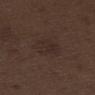Q: Was a biopsy performed?
A: imaged on a skin check; not biopsied
Q: How was the tile lit?
A: white-light illumination
Q: Lesion location?
A: the left thigh
Q: What is the lesion's diameter?
A: ~3.5 mm (longest diameter)
Q: What did automated image analysis measure?
A: an automated nevus-likeness rating near 0 out of 100
Q: How was this image acquired?
A: total-body-photography crop, ~15 mm field of view
Q: Patient demographics?
A: male, approximately 70 years of age